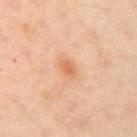Findings:
• workup · imaged on a skin check; not biopsied
• patient · female, aged 53–57
• anatomic site · the right upper arm
• acquisition · ~15 mm crop, total-body skin-cancer survey